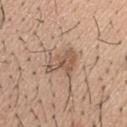{
  "biopsy_status": "not biopsied; imaged during a skin examination",
  "patient": {
    "sex": "male",
    "age_approx": 30
  },
  "image": {
    "source": "total-body photography crop",
    "field_of_view_mm": 15
  },
  "lighting": "white-light",
  "site": "head or neck"
}